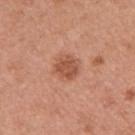Impression: This lesion was catalogued during total-body skin photography and was not selected for biopsy.